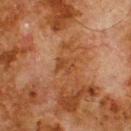notes=imaged on a skin check; not biopsied | location=the upper back | subject=male, in their 80s | TBP lesion metrics=a shape eccentricity near 0.7 and two-axis asymmetry of about 0.35; a normalized lesion–skin contrast near 5.5; border irregularity of about 3.5 on a 0–10 scale, a within-lesion color-variation index near 3/10, and a peripheral color-asymmetry measure near 1; a detector confidence of about 100 out of 100 that the crop contains a lesion | illumination=cross-polarized | acquisition=total-body-photography crop, ~15 mm field of view.